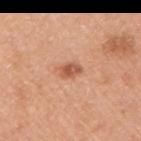| field | value |
|---|---|
| biopsy status | catalogued during a skin exam; not biopsied |
| lighting | white-light |
| lesion size | ≈2.5 mm |
| location | the right upper arm |
| subject | male, about 50 years old |
| imaging modality | 15 mm crop, total-body photography |